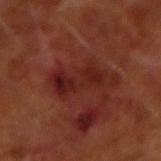biopsy_status: not biopsied; imaged during a skin examination
image:
  source: total-body photography crop
  field_of_view_mm: 15
patient:
  sex: male
  age_approx: 65
lesion_size:
  long_diameter_mm_approx: 6.5
site: right forearm
lighting: cross-polarized